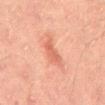No biopsy was performed on this lesion — it was imaged during a full skin examination and was not determined to be concerning. The lesion's longest dimension is about 4 mm. The patient is a male roughly 45 years of age. A roughly 15 mm field-of-view crop from a total-body skin photograph. Imaged with cross-polarized lighting. The lesion is on the mid back.